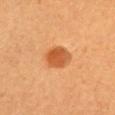{"biopsy_status": "not biopsied; imaged during a skin examination", "lighting": "cross-polarized", "patient": {"sex": "female", "age_approx": 30}, "lesion_size": {"long_diameter_mm_approx": 3.5}, "site": "chest", "automated_metrics": {"area_mm2_approx": 7.5, "eccentricity": 0.6, "shape_asymmetry": 0.15, "border_irregularity_0_10": 1.5, "color_variation_0_10": 2.5, "nevus_likeness_0_100": 100}, "image": {"source": "total-body photography crop", "field_of_view_mm": 15}}